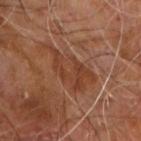workup: catalogued during a skin exam; not biopsied | diameter: about 7 mm | image source: ~15 mm tile from a whole-body skin photo | location: the upper back | automated metrics: a classifier nevus-likeness of about 0/100 and a detector confidence of about 75 out of 100 that the crop contains a lesion | subject: male, approximately 60 years of age.